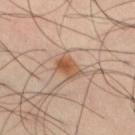workup = total-body-photography surveillance lesion; no biopsy | imaging modality = ~15 mm tile from a whole-body skin photo | tile lighting = cross-polarized illumination | anatomic site = the leg | lesion diameter = ~3.5 mm (longest diameter) | TBP lesion metrics = a lesion–skin lightness drop of about 11 and a normalized lesion–skin contrast near 8.5; a border-irregularity rating of about 3/10, a color-variation rating of about 6/10, and radial color variation of about 2 | patient = male, aged 38 to 42.